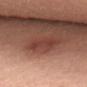follow-up — no biopsy performed (imaged during a skin exam)
location — the lower back
patient — male, aged 48 to 52
image — ~15 mm crop, total-body skin-cancer survey